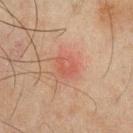Case summary:
- notes: imaged on a skin check; not biopsied
- patient: male, roughly 45 years of age
- lighting: cross-polarized illumination
- TBP lesion metrics: radial color variation of about 1.5
- body site: the chest
- diameter: ~2.5 mm (longest diameter)
- image source: 15 mm crop, total-body photography